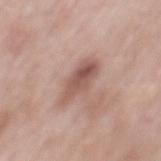Impression:
The lesion was tiled from a total-body skin photograph and was not biopsied.
Image and clinical context:
Measured at roughly 5 mm in maximum diameter. A male patient roughly 55 years of age. Imaged with white-light lighting. A 15 mm crop from a total-body photograph taken for skin-cancer surveillance. Automated image analysis of the tile measured a lesion area of about 8 mm², an outline eccentricity of about 0.9 (0 = round, 1 = elongated), and a shape-asymmetry score of about 0.25 (0 = symmetric). The software also gave a lesion color around L≈54 a*≈20 b*≈24 in CIELAB and a normalized lesion–skin contrast near 8. The lesion is on the back.